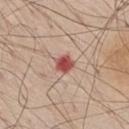Recorded during total-body skin imaging; not selected for excision or biopsy. Cropped from a whole-body photographic skin survey; the tile spans about 15 mm. A male patient, aged 63–67. The lesion is located on the right thigh. An algorithmic analysis of the crop reported a lesion area of about 4.5 mm². It also reported border irregularity of about 1.5 on a 0–10 scale, a within-lesion color-variation index near 3/10, and a peripheral color-asymmetry measure near 1. Longest diameter approximately 2.5 mm.